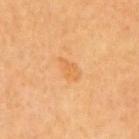Q: Was this lesion biopsied?
A: total-body-photography surveillance lesion; no biopsy